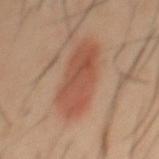Impression:
Recorded during total-body skin imaging; not selected for excision or biopsy.
Clinical summary:
On the back. A 15 mm close-up tile from a total-body photography series done for melanoma screening. The lesion-visualizer software estimated roughly 9 lightness units darker than nearby skin and a normalized lesion–skin contrast near 7. It also reported a border-irregularity rating of about 2/10, a within-lesion color-variation index near 3.5/10, and radial color variation of about 1.5. It also reported a classifier nevus-likeness of about 100/100 and a detector confidence of about 100 out of 100 that the crop contains a lesion. Imaged with cross-polarized lighting. The subject is a male aged approximately 40.